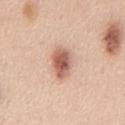Q: Is there a histopathology result?
A: total-body-photography surveillance lesion; no biopsy
Q: Automated lesion metrics?
A: an area of roughly 8.5 mm², an eccentricity of roughly 0.8, and a shape-asymmetry score of about 0.15 (0 = symmetric); a nevus-likeness score of about 95/100
Q: What is the anatomic site?
A: the chest
Q: Lesion size?
A: ≈4.5 mm
Q: Illumination type?
A: white-light
Q: Who is the patient?
A: male, in their mid- to late 40s
Q: What is the imaging modality?
A: total-body-photography crop, ~15 mm field of view